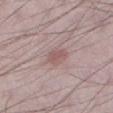Notes:
- follow-up — imaged on a skin check; not biopsied
- subject — male, in their mid-20s
- imaging modality — ~15 mm tile from a whole-body skin photo
- size — ≈3 mm
- location — the left lower leg
- image-analysis metrics — an area of roughly 4.5 mm² and a shape-asymmetry score of about 0.2 (0 = symmetric); a lesion color around L≈55 a*≈19 b*≈20 in CIELAB, a lesion–skin lightness drop of about 8, and a normalized lesion–skin contrast near 6; an automated nevus-likeness rating near 10 out of 100